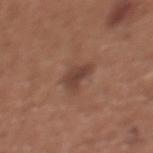Acquisition and patient details:
A lesion tile, about 15 mm wide, cut from a 3D total-body photograph. Imaged with white-light lighting. A female patient aged 33–37. The lesion is on the front of the torso.